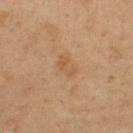Q: What did automated image analysis measure?
A: a border-irregularity rating of about 3/10, a color-variation rating of about 3/10, and peripheral color asymmetry of about 1
Q: Where on the body is the lesion?
A: the upper back
Q: How was the tile lit?
A: cross-polarized
Q: Patient demographics?
A: male, aged approximately 40
Q: What kind of image is this?
A: 15 mm crop, total-body photography